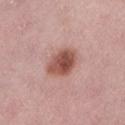Assessment:
Imaged during a routine full-body skin examination; the lesion was not biopsied and no histopathology is available.
Context:
The lesion's longest dimension is about 4 mm. From the left lower leg. Captured under white-light illumination. The total-body-photography lesion software estimated a footprint of about 10 mm². And it measured a mean CIELAB color near L≈52 a*≈24 b*≈26, about 14 CIELAB-L* units darker than the surrounding skin, and a lesion-to-skin contrast of about 10 (normalized; higher = more distinct). And it measured a border-irregularity rating of about 1.5/10, internal color variation of about 5 on a 0–10 scale, and a peripheral color-asymmetry measure near 1.5. A female subject about 50 years old. A 15 mm close-up tile from a total-body photography series done for melanoma screening.